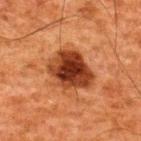Case summary:
• workup — catalogued during a skin exam; not biopsied
• site — the upper back
• lighting — cross-polarized illumination
• lesion diameter — ≈5 mm
• imaging modality — total-body-photography crop, ~15 mm field of view
• image-analysis metrics — a mean CIELAB color near L≈30 a*≈25 b*≈30, roughly 16 lightness units darker than nearby skin, and a normalized lesion–skin contrast near 13.5; a color-variation rating of about 6/10 and a peripheral color-asymmetry measure near 1.5
• subject — male, aged around 60